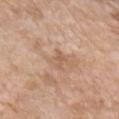follow-up: catalogued during a skin exam; not biopsied | body site: the left upper arm | lesion diameter: ≈2.5 mm | TBP lesion metrics: an eccentricity of roughly 0.9 and a symmetry-axis asymmetry near 0.45; a lesion color around L≈58 a*≈19 b*≈32 in CIELAB, a lesion–skin lightness drop of about 7, and a lesion-to-skin contrast of about 5.5 (normalized; higher = more distinct); a border-irregularity index near 5/10 and internal color variation of about 0 on a 0–10 scale; a nevus-likeness score of about 0/100 and lesion-presence confidence of about 100/100 | image source: total-body-photography crop, ~15 mm field of view | lighting: white-light | patient: female, aged around 75.